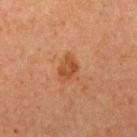Captured during whole-body skin photography for melanoma surveillance; the lesion was not biopsied.
A female patient, aged 58 to 62.
On the right upper arm.
The total-body-photography lesion software estimated an eccentricity of roughly 0.65 and two-axis asymmetry of about 0.35. It also reported a border-irregularity index near 3/10, a color-variation rating of about 2.5/10, and a peripheral color-asymmetry measure near 0.5. The analysis additionally found an automated nevus-likeness rating near 50 out of 100 and a detector confidence of about 100 out of 100 that the crop contains a lesion.
A region of skin cropped from a whole-body photographic capture, roughly 15 mm wide.
The lesion's longest dimension is about 3 mm.
Captured under cross-polarized illumination.